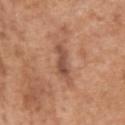workup = no biopsy performed (imaged during a skin exam)
body site = the upper back
diameter = about 4 mm
subject = male, in their mid-60s
image source = total-body-photography crop, ~15 mm field of view